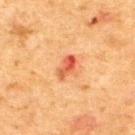{"biopsy_status": "not biopsied; imaged during a skin examination", "lighting": "cross-polarized", "automated_metrics": {"area_mm2_approx": 4.5, "eccentricity": 0.85}, "image": {"source": "total-body photography crop", "field_of_view_mm": 15}, "patient": {"sex": "male", "age_approx": 50}, "lesion_size": {"long_diameter_mm_approx": 3.5}, "site": "upper back"}